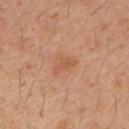<lesion>
  <biopsy_status>not biopsied; imaged during a skin examination</biopsy_status>
  <image>
    <source>total-body photography crop</source>
    <field_of_view_mm>15</field_of_view_mm>
  </image>
  <patient>
    <sex>male</sex>
    <age_approx>30</age_approx>
  </patient>
  <site>mid back</site>
  <lighting>cross-polarized</lighting>
  <automated_metrics>
    <area_mm2_approx>3.0</area_mm2_approx>
    <eccentricity>0.85</eccentricity>
    <shape_asymmetry>0.4</shape_asymmetry>
    <cielab_L>53</cielab_L>
    <cielab_a>23</cielab_a>
    <cielab_b>34</cielab_b>
    <vs_skin_darker_L>6.0</vs_skin_darker_L>
    <vs_skin_contrast_norm>5.0</vs_skin_contrast_norm>
    <nevus_likeness_0_100>15</nevus_likeness_0_100>
    <lesion_detection_confidence_0_100>100</lesion_detection_confidence_0_100>
  </automated_metrics>
</lesion>